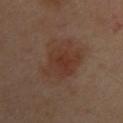workup: imaged on a skin check; not biopsied | image source: ~15 mm crop, total-body skin-cancer survey | patient: female, roughly 40 years of age | anatomic site: the upper back.